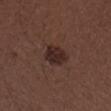• subject — male, in their 30s
• image source — total-body-photography crop, ~15 mm field of view
• lighting — white-light
• body site — the left forearm